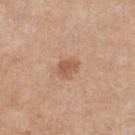Recorded during total-body skin imaging; not selected for excision or biopsy.
Located on the left lower leg.
Cropped from a total-body skin-imaging series; the visible field is about 15 mm.
The total-body-photography lesion software estimated a footprint of about 4 mm² and two-axis asymmetry of about 0.3. The analysis additionally found a lesion color around L≈56 a*≈22 b*≈32 in CIELAB, about 9 CIELAB-L* units darker than the surrounding skin, and a normalized lesion–skin contrast near 6.5. And it measured a nevus-likeness score of about 50/100.
The lesion's longest dimension is about 2.5 mm.
The tile uses white-light illumination.
A female patient, roughly 45 years of age.